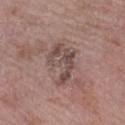Clinical impression: Part of a total-body skin-imaging series; this lesion was reviewed on a skin check and was not flagged for biopsy. Context: The recorded lesion diameter is about 5.5 mm. On the left lower leg. A lesion tile, about 15 mm wide, cut from a 3D total-body photograph. A female patient, in their mid- to late 80s or older.